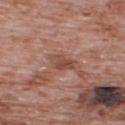Q: Was a biopsy performed?
A: total-body-photography surveillance lesion; no biopsy
Q: Who is the patient?
A: male, aged 68 to 72
Q: What is the anatomic site?
A: the upper back
Q: Illumination type?
A: white-light
Q: Lesion size?
A: ~3 mm (longest diameter)
Q: What did automated image analysis measure?
A: a lesion area of about 5 mm², an outline eccentricity of about 0.7 (0 = round, 1 = elongated), and a symmetry-axis asymmetry near 0.3; a lesion color around L≈48 a*≈22 b*≈26 in CIELAB, a lesion–skin lightness drop of about 9, and a normalized lesion–skin contrast near 6.5
Q: What is the imaging modality?
A: total-body-photography crop, ~15 mm field of view